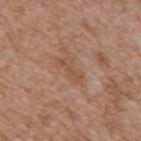Part of a total-body skin-imaging series; this lesion was reviewed on a skin check and was not flagged for biopsy. A male subject roughly 65 years of age. A 15 mm crop from a total-body photograph taken for skin-cancer surveillance. On the mid back.